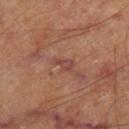Clinical impression:
Part of a total-body skin-imaging series; this lesion was reviewed on a skin check and was not flagged for biopsy.
Background:
The lesion is located on the right thigh. This is a cross-polarized tile. The subject is a male aged 68 to 72. A 15 mm close-up tile from a total-body photography series done for melanoma screening. The recorded lesion diameter is about 2.5 mm.